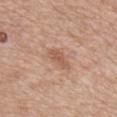Findings:
* follow-up — no biopsy performed (imaged during a skin exam)
* illumination — white-light
* anatomic site — the back
* TBP lesion metrics — an average lesion color of about L≈56 a*≈22 b*≈30 (CIELAB) and a normalized lesion–skin contrast near 6; border irregularity of about 3 on a 0–10 scale, a within-lesion color-variation index near 1/10, and peripheral color asymmetry of about 0.5
* lesion diameter — about 4 mm
* acquisition — total-body-photography crop, ~15 mm field of view
* subject — male, approximately 80 years of age